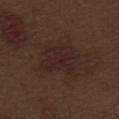Recorded during total-body skin imaging; not selected for excision or biopsy.
A region of skin cropped from a whole-body photographic capture, roughly 15 mm wide.
The lesion is located on the abdomen.
A male subject, aged around 70.